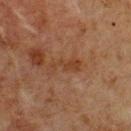Assessment:
Imaged during a routine full-body skin examination; the lesion was not biopsied and no histopathology is available.
Clinical summary:
The subject is a male aged 73–77. Automated image analysis of the tile measured an area of roughly 5 mm², an outline eccentricity of about 0.95 (0 = round, 1 = elongated), and a symmetry-axis asymmetry near 0.4. And it measured a mean CIELAB color near L≈32 a*≈18 b*≈27, roughly 5 lightness units darker than nearby skin, and a normalized lesion–skin contrast near 6. The software also gave a lesion-detection confidence of about 100/100. This is a cross-polarized tile. The lesion's longest dimension is about 4 mm. On the chest. A lesion tile, about 15 mm wide, cut from a 3D total-body photograph.